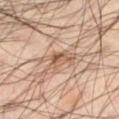Q: Was this lesion biopsied?
A: imaged on a skin check; not biopsied
Q: Where on the body is the lesion?
A: the leg
Q: What is the lesion's diameter?
A: ≈4.5 mm
Q: What did automated image analysis measure?
A: a shape eccentricity near 0.85 and a symmetry-axis asymmetry near 0.45; an average lesion color of about L≈55 a*≈17 b*≈29 (CIELAB), a lesion–skin lightness drop of about 10, and a lesion-to-skin contrast of about 7 (normalized; higher = more distinct)
Q: What kind of image is this?
A: ~15 mm tile from a whole-body skin photo
Q: What lighting was used for the tile?
A: cross-polarized illumination
Q: Who is the patient?
A: male, in their mid-60s